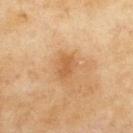Imaged during a routine full-body skin examination; the lesion was not biopsied and no histopathology is available. A female subject, aged 58 to 62. From the upper back. Imaged with cross-polarized lighting. An algorithmic analysis of the crop reported a footprint of about 4.5 mm² and an outline eccentricity of about 0.8 (0 = round, 1 = elongated). The software also gave roughly 8 lightness units darker than nearby skin and a lesion-to-skin contrast of about 6 (normalized; higher = more distinct). It also reported a border-irregularity rating of about 2/10, a within-lesion color-variation index near 2/10, and radial color variation of about 1. The analysis additionally found an automated nevus-likeness rating near 5 out of 100 and lesion-presence confidence of about 100/100. This image is a 15 mm lesion crop taken from a total-body photograph.